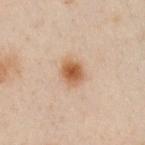The lesion was photographed on a routine skin check and not biopsied; there is no pathology result.
The recorded lesion diameter is about 3 mm.
A lesion tile, about 15 mm wide, cut from a 3D total-body photograph.
Imaged with cross-polarized lighting.
Automated tile analysis of the lesion measured an area of roughly 5.5 mm² and a symmetry-axis asymmetry near 0.2. It also reported a classifier nevus-likeness of about 100/100 and a lesion-detection confidence of about 100/100.
The lesion is located on the left arm.
A male patient, aged 48 to 52.